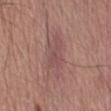Case summary:
* workup: catalogued during a skin exam; not biopsied
* automated lesion analysis: a mean CIELAB color near L≈50 a*≈21 b*≈20, about 7 CIELAB-L* units darker than the surrounding skin, and a lesion-to-skin contrast of about 5.5 (normalized; higher = more distinct)
* image source: ~15 mm tile from a whole-body skin photo
* patient: male, aged around 55
* lesion diameter: ~6 mm (longest diameter)